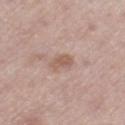  biopsy_status: not biopsied; imaged during a skin examination
  patient:
    sex: male
    age_approx: 75
  lighting: white-light
  automated_metrics:
    area_mm2_approx: 4.0
    shape_asymmetry: 0.3
    vs_skin_darker_L: 9.0
  lesion_size:
    long_diameter_mm_approx: 2.5
  site: left thigh
  image:
    source: total-body photography crop
    field_of_view_mm: 15